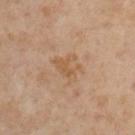Recorded during total-body skin imaging; not selected for excision or biopsy. A lesion tile, about 15 mm wide, cut from a 3D total-body photograph. Approximately 3.5 mm at its widest. On the right upper arm. A male patient, roughly 55 years of age. The tile uses cross-polarized illumination.